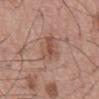The lesion was tiled from a total-body skin photograph and was not biopsied. On the back. A male patient, approximately 55 years of age. A roughly 15 mm field-of-view crop from a total-body skin photograph. The tile uses white-light illumination.